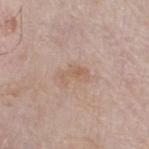{
  "biopsy_status": "not biopsied; imaged during a skin examination",
  "image": {
    "source": "total-body photography crop",
    "field_of_view_mm": 15
  },
  "lesion_size": {
    "long_diameter_mm_approx": 3.0
  },
  "lighting": "white-light",
  "site": "left lower leg",
  "patient": {
    "sex": "male",
    "age_approx": 80
  }
}